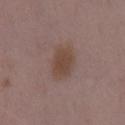Captured during whole-body skin photography for melanoma surveillance; the lesion was not biopsied. Located on the mid back. Approximately 4.5 mm at its widest. A lesion tile, about 15 mm wide, cut from a 3D total-body photograph. A female patient, in their mid- to late 30s. The tile uses white-light illumination.